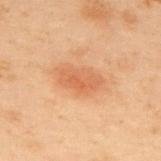Findings:
– notes — total-body-photography surveillance lesion; no biopsy
– subject — male, roughly 55 years of age
– illumination — cross-polarized
– image source — ~15 mm crop, total-body skin-cancer survey
– body site — the upper back
– diameter — ≈4.5 mm